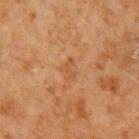follow-up: total-body-photography surveillance lesion; no biopsy
lighting: cross-polarized
image: total-body-photography crop, ~15 mm field of view
anatomic site: the arm
automated lesion analysis: an eccentricity of roughly 0.85 and a shape-asymmetry score of about 0.5 (0 = symmetric); a border-irregularity index near 5.5/10, a color-variation rating of about 0/10, and radial color variation of about 0; a nevus-likeness score of about 0/100 and lesion-presence confidence of about 100/100
patient: male, aged around 60